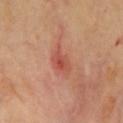This lesion was catalogued during total-body skin photography and was not selected for biopsy.
From the chest.
This is a cross-polarized tile.
The total-body-photography lesion software estimated an area of roughly 6.5 mm², an outline eccentricity of about 0.85 (0 = round, 1 = elongated), and two-axis asymmetry of about 0.3. And it measured a lesion color around L≈53 a*≈30 b*≈32 in CIELAB, roughly 9 lightness units darker than nearby skin, and a lesion-to-skin contrast of about 6.5 (normalized; higher = more distinct). The analysis additionally found a border-irregularity index near 3/10, a color-variation rating of about 5.5/10, and peripheral color asymmetry of about 1.5. The software also gave an automated nevus-likeness rating near 0 out of 100 and lesion-presence confidence of about 100/100.
This image is a 15 mm lesion crop taken from a total-body photograph.
A female patient, roughly 60 years of age.
About 4 mm across.